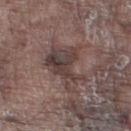This is a white-light tile.
The lesion is on the right thigh.
A male patient, aged 73–77.
Longest diameter approximately 7.5 mm.
A 15 mm close-up tile from a total-body photography series done for melanoma screening.